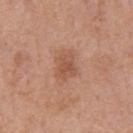  biopsy_status: not biopsied; imaged during a skin examination
  lighting: white-light
  lesion_size:
    long_diameter_mm_approx: 3.0
  automated_metrics:
    area_mm2_approx: 5.5
    eccentricity: 0.65
    shape_asymmetry: 0.3
    nevus_likeness_0_100: 20
  patient:
    sex: male
    age_approx: 70
  image:
    source: total-body photography crop
    field_of_view_mm: 15
  site: chest